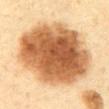{
  "biopsy_status": "not biopsied; imaged during a skin examination",
  "lighting": "cross-polarized",
  "site": "abdomen",
  "image": {
    "source": "total-body photography crop",
    "field_of_view_mm": 15
  },
  "lesion_size": {
    "long_diameter_mm_approx": 10.0
  },
  "patient": {
    "sex": "male",
    "age_approx": 60
  }
}